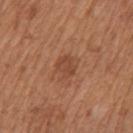biopsy_status: not biopsied; imaged during a skin examination
site: mid back
image:
  source: total-body photography crop
  field_of_view_mm: 15
automated_metrics:
  area_mm2_approx: 4.0
  eccentricity: 0.6
  cielab_L: 46
  cielab_a: 24
  cielab_b: 32
  vs_skin_darker_L: 7.0
  border_irregularity_0_10: 2.5
  color_variation_0_10: 2.0
  peripheral_color_asymmetry: 0.5
  nevus_likeness_0_100: 0
  lesion_detection_confidence_0_100: 100
patient:
  sex: male
  age_approx: 65
lesion_size:
  long_diameter_mm_approx: 2.5
lighting: white-light